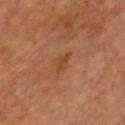Findings:
– anatomic site · the chest
– image-analysis metrics · an automated nevus-likeness rating near 0 out of 100 and a lesion-detection confidence of about 100/100
– patient · male, aged around 65
– image source · ~15 mm tile from a whole-body skin photo
– lesion diameter · ≈3 mm
– tile lighting · cross-polarized illumination